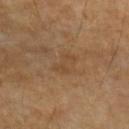Recorded during total-body skin imaging; not selected for excision or biopsy. About 3 mm across. The total-body-photography lesion software estimated a lesion color around L≈43 a*≈17 b*≈32 in CIELAB, roughly 5 lightness units darker than nearby skin, and a lesion-to-skin contrast of about 4.5 (normalized; higher = more distinct). It also reported border irregularity of about 6.5 on a 0–10 scale and a within-lesion color-variation index near 0.5/10. And it measured a classifier nevus-likeness of about 0/100. From the left forearm. This is a cross-polarized tile. This image is a 15 mm lesion crop taken from a total-body photograph. A male subject aged approximately 85 or older.